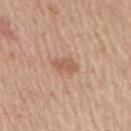No biopsy was performed on this lesion — it was imaged during a full skin examination and was not determined to be concerning. A region of skin cropped from a whole-body photographic capture, roughly 15 mm wide. The patient is a male aged 73–77. Approximately 3 mm at its widest. Automated image analysis of the tile measured an area of roughly 4.5 mm², an eccentricity of roughly 0.8, and a shape-asymmetry score of about 0.2 (0 = symmetric). The analysis additionally found a lesion–skin lightness drop of about 9 and a lesion-to-skin contrast of about 6 (normalized; higher = more distinct). The analysis additionally found a border-irregularity rating of about 2.5/10 and radial color variation of about 0.5. From the right upper arm.